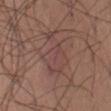biopsy_status: not biopsied; imaged during a skin examination
lighting: white-light
patient:
  sex: male
  age_approx: 25
image:
  source: total-body photography crop
  field_of_view_mm: 15
automated_metrics:
  area_mm2_approx: 14.0
  shape_asymmetry: 0.45
  cielab_L: 44
  cielab_a: 19
  cielab_b: 21
  vs_skin_contrast_norm: 5.0
  nevus_likeness_0_100: 0
  lesion_detection_confidence_0_100: 95
site: abdomen
lesion_size:
  long_diameter_mm_approx: 6.0